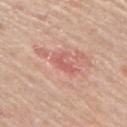biopsy status = imaged on a skin check; not biopsied | anatomic site = the right upper arm | patient = female, aged 68–72 | image source = ~15 mm tile from a whole-body skin photo.